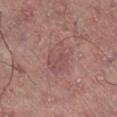<lesion>
<biopsy_status>not biopsied; imaged during a skin examination</biopsy_status>
<lesion_size>
  <long_diameter_mm_approx>4.5</long_diameter_mm_approx>
</lesion_size>
<site>leg</site>
<automated_metrics>
  <area_mm2_approx>9.5</area_mm2_approx>
  <eccentricity>0.75</eccentricity>
  <shape_asymmetry>0.2</shape_asymmetry>
  <cielab_L>43</cielab_L>
  <cielab_a>20</cielab_a>
  <cielab_b>18</cielab_b>
  <vs_skin_contrast_norm>5.0</vs_skin_contrast_norm>
  <nevus_likeness_0_100>0</nevus_likeness_0_100>
  <lesion_detection_confidence_0_100>100</lesion_detection_confidence_0_100>
</automated_metrics>
<image>
  <source>total-body photography crop</source>
  <field_of_view_mm>15</field_of_view_mm>
</image>
<lighting>cross-polarized</lighting>
<patient>
  <sex>male</sex>
  <age_approx>60</age_approx>
</patient>
</lesion>